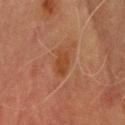The lesion was tiled from a total-body skin photograph and was not biopsied. Located on the head or neck. This is a cross-polarized tile. The subject is a male about 70 years old. About 3.5 mm across. The lesion-visualizer software estimated an average lesion color of about L≈36 a*≈21 b*≈31 (CIELAB). The software also gave a border-irregularity index near 2/10, a within-lesion color-variation index near 1.5/10, and radial color variation of about 0.5. And it measured an automated nevus-likeness rating near 5 out of 100 and lesion-presence confidence of about 100/100. A 15 mm crop from a total-body photograph taken for skin-cancer surveillance.